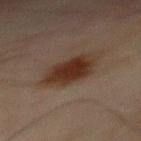Q: Was this lesion biopsied?
A: no biopsy performed (imaged during a skin exam)
Q: How was this image acquired?
A: ~15 mm tile from a whole-body skin photo
Q: What are the patient's age and sex?
A: male, aged 68–72
Q: Where on the body is the lesion?
A: the front of the torso